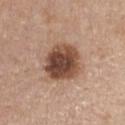Part of a total-body skin-imaging series; this lesion was reviewed on a skin check and was not flagged for biopsy.
About 5 mm across.
The lesion is on the chest.
A 15 mm crop from a total-body photograph taken for skin-cancer surveillance.
A female subject approximately 45 years of age.
Imaged with white-light lighting.